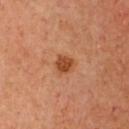imaging modality = ~15 mm crop, total-body skin-cancer survey; site = the chest; lighting = cross-polarized; lesion size = ~2.5 mm (longest diameter); patient = female, aged around 40.